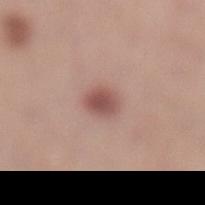workup: catalogued during a skin exam; not biopsied | diameter: ≈2.5 mm | image source: ~15 mm tile from a whole-body skin photo | anatomic site: the left lower leg | patient: female, aged approximately 30 | TBP lesion metrics: an area of roughly 5 mm² and two-axis asymmetry of about 0.1.